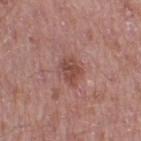Clinical impression: Captured during whole-body skin photography for melanoma surveillance; the lesion was not biopsied. Clinical summary: The lesion is located on the right thigh. The subject is a male aged 48 to 52. A lesion tile, about 15 mm wide, cut from a 3D total-body photograph.